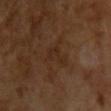Assessment: Imaged during a routine full-body skin examination; the lesion was not biopsied and no histopathology is available. Context: This image is a 15 mm lesion crop taken from a total-body photograph. The subject is a male in their mid- to late 60s. Imaged with cross-polarized lighting. Measured at roughly 3.5 mm in maximum diameter.